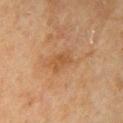notes = total-body-photography surveillance lesion; no biopsy | image source = total-body-photography crop, ~15 mm field of view | lighting = cross-polarized | lesion size = ~2.5 mm (longest diameter) | location = the arm | patient = female, aged approximately 80.